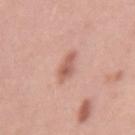Captured during whole-body skin photography for melanoma surveillance; the lesion was not biopsied. The recorded lesion diameter is about 3.5 mm. A female subject, in their mid-30s. From the left upper arm. A 15 mm close-up extracted from a 3D total-body photography capture. The tile uses white-light illumination.